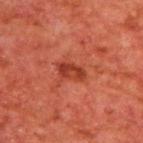Clinical impression: Recorded during total-body skin imaging; not selected for excision or biopsy. Acquisition and patient details: Measured at roughly 3 mm in maximum diameter. A male subject, aged 63 to 67. This is a cross-polarized tile. The lesion is on the upper back. Cropped from a total-body skin-imaging series; the visible field is about 15 mm.